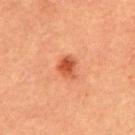Q: Was a biopsy performed?
A: imaged on a skin check; not biopsied
Q: How was this image acquired?
A: total-body-photography crop, ~15 mm field of view
Q: Patient demographics?
A: male, in their mid-60s
Q: What is the anatomic site?
A: the abdomen
Q: Automated lesion metrics?
A: a lesion area of about 5 mm², a shape eccentricity near 0.65, and two-axis asymmetry of about 0.3
Q: How large is the lesion?
A: about 2.5 mm